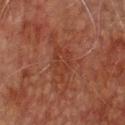Findings:
- notes — imaged on a skin check; not biopsied
- body site — the chest
- subject — male, roughly 75 years of age
- image source — 15 mm crop, total-body photography